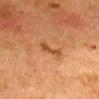Recorded during total-body skin imaging; not selected for excision or biopsy. An algorithmic analysis of the crop reported a lesion color around L≈41 a*≈21 b*≈36 in CIELAB. The software also gave a classifier nevus-likeness of about 0/100 and a detector confidence of about 100 out of 100 that the crop contains a lesion. Cropped from a total-body skin-imaging series; the visible field is about 15 mm. Located on the front of the torso. Captured under cross-polarized illumination. A female subject, approximately 50 years of age. Longest diameter approximately 3 mm.